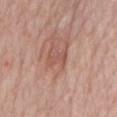The lesion was photographed on a routine skin check and not biopsied; there is no pathology result.
A 15 mm crop from a total-body photograph taken for skin-cancer surveillance.
Located on the mid back.
Measured at roughly 2.5 mm in maximum diameter.
A male patient in their mid-70s.
Automated image analysis of the tile measured a footprint of about 3.5 mm², a shape eccentricity near 0.8, and two-axis asymmetry of about 0.55. The analysis additionally found a within-lesion color-variation index near 0.5/10 and radial color variation of about 0.5.